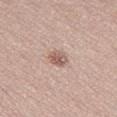Captured during whole-body skin photography for melanoma surveillance; the lesion was not biopsied. Approximately 2.5 mm at its widest. A 15 mm close-up extracted from a 3D total-body photography capture. The subject is a female aged approximately 25. From the leg. Automated image analysis of the tile measured a border-irregularity index near 2/10 and internal color variation of about 4 on a 0–10 scale. The software also gave an automated nevus-likeness rating near 65 out of 100 and a lesion-detection confidence of about 100/100.